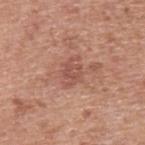<lesion>
<biopsy_status>not biopsied; imaged during a skin examination</biopsy_status>
<site>upper back</site>
<image>
  <source>total-body photography crop</source>
  <field_of_view_mm>15</field_of_view_mm>
</image>
<patient>
  <sex>male</sex>
  <age_approx>55</age_approx>
</patient>
</lesion>